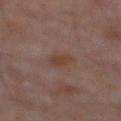Findings:
- body site · the abdomen
- tile lighting · cross-polarized
- lesion size · about 2.5 mm
- patient · male, aged approximately 60
- imaging modality · ~15 mm crop, total-body skin-cancer survey